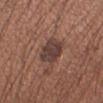<case>
  <biopsy_status>not biopsied; imaged during a skin examination</biopsy_status>
  <patient>
    <sex>male</sex>
    <age_approx>35</age_approx>
  </patient>
  <lesion_size>
    <long_diameter_mm_approx>3.5</long_diameter_mm_approx>
  </lesion_size>
  <automated_metrics>
    <cielab_L>39</cielab_L>
    <cielab_a>18</cielab_a>
    <cielab_b>21</cielab_b>
    <vs_skin_darker_L>11.0</vs_skin_darker_L>
    <border_irregularity_0_10>2.0</border_irregularity_0_10>
    <color_variation_0_10>3.5</color_variation_0_10>
    <peripheral_color_asymmetry>1.0</peripheral_color_asymmetry>
  </automated_metrics>
  <image>
    <source>total-body photography crop</source>
    <field_of_view_mm>15</field_of_view_mm>
  </image>
  <site>left upper arm</site>
</case>